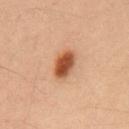This lesion was catalogued during total-body skin photography and was not selected for biopsy. A roughly 15 mm field-of-view crop from a total-body skin photograph. A male subject in their mid-30s. From the chest.